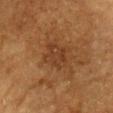The lesion was photographed on a routine skin check and not biopsied; there is no pathology result. A lesion tile, about 15 mm wide, cut from a 3D total-body photograph. From the head or neck. The tile uses cross-polarized illumination. The lesion-visualizer software estimated a lesion area of about 8 mm², an eccentricity of roughly 0.65, and a shape-asymmetry score of about 0.25 (0 = symmetric). And it measured a mean CIELAB color near L≈33 a*≈19 b*≈30, a lesion–skin lightness drop of about 6, and a normalized border contrast of about 5.5. And it measured a border-irregularity index near 3/10, a within-lesion color-variation index near 2.5/10, and a peripheral color-asymmetry measure near 1. The analysis additionally found an automated nevus-likeness rating near 0 out of 100 and lesion-presence confidence of about 100/100. The patient is a male aged around 85 or older.